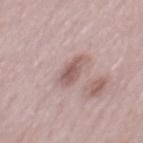| feature | finding |
|---|---|
| biopsy status | no biopsy performed (imaged during a skin exam) |
| lighting | white-light |
| imaging modality | ~15 mm crop, total-body skin-cancer survey |
| patient | male, aged around 50 |
| TBP lesion metrics | a lesion area of about 6 mm², an outline eccentricity of about 0.9 (0 = round, 1 = elongated), and two-axis asymmetry of about 0.3; a detector confidence of about 95 out of 100 that the crop contains a lesion |
| location | the mid back |